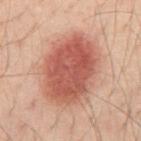Clinical impression:
Imaged during a routine full-body skin examination; the lesion was not biopsied and no histopathology is available.
Image and clinical context:
Located on the abdomen. A male subject aged around 40. A 15 mm crop from a total-body photograph taken for skin-cancer surveillance.